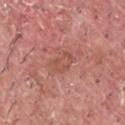  biopsy_status: not biopsied; imaged during a skin examination
  automated_metrics:
    area_mm2_approx: 5.5
    eccentricity: 0.75
    shape_asymmetry: 0.3
    cielab_L: 52
    cielab_a: 26
    cielab_b: 28
    vs_skin_contrast_norm: 5.0
    nevus_likeness_0_100: 0
  lighting: white-light
  image:
    source: total-body photography crop
    field_of_view_mm: 15
  patient:
    sex: male
    age_approx: 40
  site: left upper arm